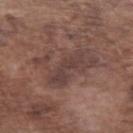<tbp_lesion>
  <biopsy_status>not biopsied; imaged during a skin examination</biopsy_status>
  <image>
    <source>total-body photography crop</source>
    <field_of_view_mm>15</field_of_view_mm>
  </image>
  <site>left forearm</site>
  <lighting>white-light</lighting>
  <lesion_size>
    <long_diameter_mm_approx>6.5</long_diameter_mm_approx>
  </lesion_size>
  <patient>
    <sex>male</sex>
    <age_approx>75</age_approx>
  </patient>
</tbp_lesion>